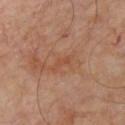{"biopsy_status": "not biopsied; imaged during a skin examination", "site": "chest", "image": {"source": "total-body photography crop", "field_of_view_mm": 15}, "patient": {"sex": "male", "age_approx": 55}, "lighting": "cross-polarized", "lesion_size": {"long_diameter_mm_approx": 2.5}}